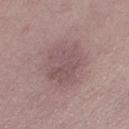Q: Was a biopsy performed?
A: total-body-photography surveillance lesion; no biopsy
Q: What is the anatomic site?
A: the leg
Q: What are the patient's age and sex?
A: female, aged 58–62
Q: What lighting was used for the tile?
A: white-light illumination
Q: What is the lesion's diameter?
A: ≈6 mm
Q: What kind of image is this?
A: ~15 mm crop, total-body skin-cancer survey